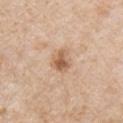notes = imaged on a skin check; not biopsied
subject = male, aged approximately 65
lesion diameter = ~3 mm (longest diameter)
body site = the chest
tile lighting = white-light illumination
acquisition = ~15 mm crop, total-body skin-cancer survey